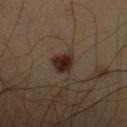| feature | finding |
|---|---|
| biopsy status | total-body-photography surveillance lesion; no biopsy |
| subject | male, roughly 35 years of age |
| lesion diameter | ≈2.5 mm |
| image | 15 mm crop, total-body photography |
| anatomic site | the left upper arm |
| lighting | cross-polarized |